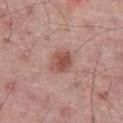This lesion was catalogued during total-body skin photography and was not selected for biopsy.
Cropped from a whole-body photographic skin survey; the tile spans about 15 mm.
Captured under white-light illumination.
A male patient, approximately 65 years of age.
Longest diameter approximately 3 mm.
The lesion is located on the front of the torso.